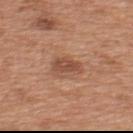Q: Is there a histopathology result?
A: no biopsy performed (imaged during a skin exam)
Q: Patient demographics?
A: male, in their mid-60s
Q: What did automated image analysis measure?
A: a mean CIELAB color near L≈50 a*≈23 b*≈31, a lesion–skin lightness drop of about 10, and a normalized border contrast of about 7; a classifier nevus-likeness of about 60/100
Q: What kind of image is this?
A: total-body-photography crop, ~15 mm field of view
Q: Lesion location?
A: the upper back